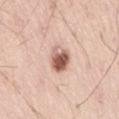Clinical impression: This lesion was catalogued during total-body skin photography and was not selected for biopsy. Image and clinical context: The subject is a male aged around 55. Located on the lower back. The lesion's longest dimension is about 3.5 mm. The lesion-visualizer software estimated a lesion color around L≈59 a*≈21 b*≈27 in CIELAB, a lesion–skin lightness drop of about 17, and a normalized border contrast of about 10.5. And it measured border irregularity of about 2 on a 0–10 scale, internal color variation of about 9 on a 0–10 scale, and radial color variation of about 3. A lesion tile, about 15 mm wide, cut from a 3D total-body photograph. This is a white-light tile.